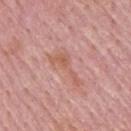notes = catalogued during a skin exam; not biopsied | image-analysis metrics = an automated nevus-likeness rating near 0 out of 100 and lesion-presence confidence of about 100/100 | acquisition = 15 mm crop, total-body photography | anatomic site = the back | patient = male, aged 63–67 | diameter = about 5 mm.